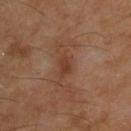Assessment:
The lesion was tiled from a total-body skin photograph and was not biopsied.
Background:
Cropped from a whole-body photographic skin survey; the tile spans about 15 mm. A male subject, roughly 55 years of age. The lesion is located on the upper back.